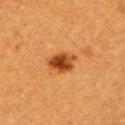Impression:
This lesion was catalogued during total-body skin photography and was not selected for biopsy.
Clinical summary:
A female patient, aged around 55. The lesion's longest dimension is about 3.5 mm. Imaged with cross-polarized lighting. A 15 mm crop from a total-body photograph taken for skin-cancer surveillance. The lesion-visualizer software estimated a symmetry-axis asymmetry near 0.25. Located on the left upper arm.